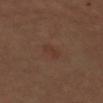– subject — female, aged approximately 70
– image source — 15 mm crop, total-body photography
– illumination — cross-polarized illumination
– location — the left upper arm